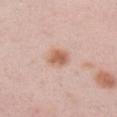The lesion was tiled from a total-body skin photograph and was not biopsied.
The patient is a male approximately 35 years of age.
Cropped from a whole-body photographic skin survey; the tile spans about 15 mm.
Located on the right upper arm.
Longest diameter approximately 3 mm.